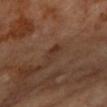Part of a total-body skin-imaging series; this lesion was reviewed on a skin check and was not flagged for biopsy. Cropped from a total-body skin-imaging series; the visible field is about 15 mm. On the left forearm. The tile uses cross-polarized illumination. The lesion's longest dimension is about 3 mm. A female patient aged approximately 60.